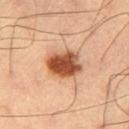The patient is a male aged approximately 60. The recorded lesion diameter is about 4 mm. The lesion-visualizer software estimated a footprint of about 11 mm². Located on the left thigh. The tile uses cross-polarized illumination. A 15 mm close-up tile from a total-body photography series done for melanoma screening.